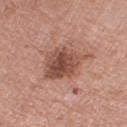The lesion was tiled from a total-body skin photograph and was not biopsied.
Captured under white-light illumination.
A female patient roughly 75 years of age.
From the right thigh.
Measured at roughly 5.5 mm in maximum diameter.
A 15 mm close-up extracted from a 3D total-body photography capture.
Automated image analysis of the tile measured a lesion area of about 15 mm², a shape eccentricity near 0.6, and two-axis asymmetry of about 0.3. The software also gave a mean CIELAB color near L≈49 a*≈23 b*≈27 and about 13 CIELAB-L* units darker than the surrounding skin. The software also gave a border-irregularity rating of about 3.5/10 and internal color variation of about 6 on a 0–10 scale. And it measured a classifier nevus-likeness of about 45/100 and a lesion-detection confidence of about 100/100.